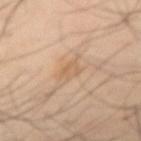Assessment: This lesion was catalogued during total-body skin photography and was not selected for biopsy. Acquisition and patient details: The subject is a male aged 38–42. From the right upper arm. A lesion tile, about 15 mm wide, cut from a 3D total-body photograph.